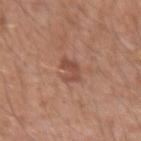Impression:
Recorded during total-body skin imaging; not selected for excision or biopsy.
Acquisition and patient details:
Captured under white-light illumination. The lesion's longest dimension is about 2.5 mm. A close-up tile cropped from a whole-body skin photograph, about 15 mm across. The lesion is on the arm. A male subject, in their 30s.